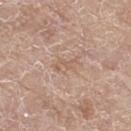Cropped from a total-body skin-imaging series; the visible field is about 15 mm. A male subject about 80 years old. Automated image analysis of the tile measured a footprint of about 3.5 mm² and a symmetry-axis asymmetry near 0.35. It also reported a border-irregularity index near 3.5/10 and peripheral color asymmetry of about 0.5. And it measured a classifier nevus-likeness of about 0/100 and a lesion-detection confidence of about 95/100. Captured under white-light illumination. Located on the right thigh.